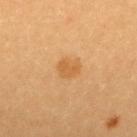workup — imaged on a skin check; not biopsied
automated lesion analysis — an area of roughly 4 mm², an outline eccentricity of about 0.6 (0 = round, 1 = elongated), and two-axis asymmetry of about 0.35; an average lesion color of about L≈59 a*≈23 b*≈45 (CIELAB), roughly 8 lightness units darker than nearby skin, and a normalized lesion–skin contrast near 6; a nevus-likeness score of about 55/100 and a lesion-detection confidence of about 100/100
acquisition — ~15 mm tile from a whole-body skin photo
location — the upper back
patient — female, aged approximately 25
lighting — cross-polarized illumination